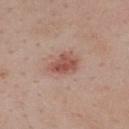Assessment:
No biopsy was performed on this lesion — it was imaged during a full skin examination and was not determined to be concerning.